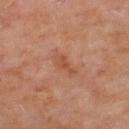Q: Is there a histopathology result?
A: catalogued during a skin exam; not biopsied
Q: How was the tile lit?
A: cross-polarized illumination
Q: What are the patient's age and sex?
A: male, aged around 60
Q: What is the anatomic site?
A: the mid back
Q: What is the imaging modality?
A: ~15 mm tile from a whole-body skin photo